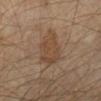The lesion was photographed on a routine skin check and not biopsied; there is no pathology result. This image is a 15 mm lesion crop taken from a total-body photograph. The patient is in their mid-50s. This is a cross-polarized tile. The lesion's longest dimension is about 4 mm. The lesion is on the left lower leg.